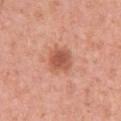Q: What kind of image is this?
A: 15 mm crop, total-body photography
Q: What are the patient's age and sex?
A: female, roughly 35 years of age
Q: Lesion location?
A: the left upper arm